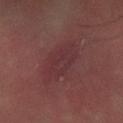patient: male, roughly 45 years of age; location: the left forearm; imaging modality: ~15 mm crop, total-body skin-cancer survey.